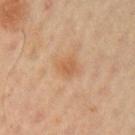| field | value |
|---|---|
| follow-up | no biopsy performed (imaged during a skin exam) |
| image-analysis metrics | an eccentricity of roughly 0.45 and two-axis asymmetry of about 0.3; about 7 CIELAB-L* units darker than the surrounding skin; border irregularity of about 2.5 on a 0–10 scale, a within-lesion color-variation index near 1.5/10, and peripheral color asymmetry of about 0.5 |
| illumination | cross-polarized illumination |
| patient | male, aged approximately 60 |
| lesion size | about 2.5 mm |
| acquisition | ~15 mm crop, total-body skin-cancer survey |
| anatomic site | the left upper arm |